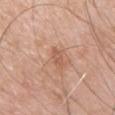notes = total-body-photography surveillance lesion; no biopsy | lesion diameter = about 3 mm | TBP lesion metrics = a footprint of about 3 mm², a shape eccentricity near 0.9, and two-axis asymmetry of about 0.2; border irregularity of about 2.5 on a 0–10 scale, internal color variation of about 2 on a 0–10 scale, and peripheral color asymmetry of about 1; a classifier nevus-likeness of about 0/100 and a lesion-detection confidence of about 100/100 | image = ~15 mm crop, total-body skin-cancer survey | body site = the chest | subject = male, in their mid-50s | tile lighting = white-light.